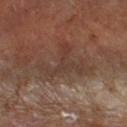Clinical impression:
No biopsy was performed on this lesion — it was imaged during a full skin examination and was not determined to be concerning.
Image and clinical context:
The lesion is located on the right forearm. A 15 mm close-up extracted from a 3D total-body photography capture. A male patient aged 63 to 67. Captured under cross-polarized illumination. An algorithmic analysis of the crop reported a shape eccentricity near 0.7 and a shape-asymmetry score of about 0.7 (0 = symmetric). It also reported an average lesion color of about L≈38 a*≈17 b*≈24 (CIELAB) and a lesion–skin lightness drop of about 6.